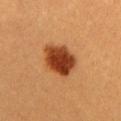Part of a total-body skin-imaging series; this lesion was reviewed on a skin check and was not flagged for biopsy.
From the chest.
Longest diameter approximately 5 mm.
A female patient approximately 30 years of age.
Imaged with cross-polarized lighting.
Cropped from a whole-body photographic skin survey; the tile spans about 15 mm.